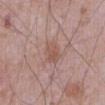<tbp_lesion>
  <lighting>white-light</lighting>
  <image>
    <source>total-body photography crop</source>
    <field_of_view_mm>15</field_of_view_mm>
  </image>
  <patient>
    <sex>male</sex>
    <age_approx>70</age_approx>
  </patient>
  <site>abdomen</site>
</tbp_lesion>